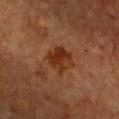Captured during whole-body skin photography for melanoma surveillance; the lesion was not biopsied. A 15 mm crop from a total-body photograph taken for skin-cancer surveillance. The lesion is on the chest. The recorded lesion diameter is about 3 mm. A female patient aged approximately 55.